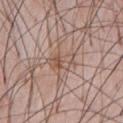Q: What did automated image analysis measure?
A: an eccentricity of roughly 0.9 and a symmetry-axis asymmetry near 0.35; a border-irregularity rating of about 4/10, a color-variation rating of about 7.5/10, and radial color variation of about 3; an automated nevus-likeness rating near 0 out of 100 and a lesion-detection confidence of about 100/100
Q: Lesion location?
A: the chest
Q: What is the lesion's diameter?
A: ≈4 mm
Q: What kind of image is this?
A: ~15 mm crop, total-body skin-cancer survey
Q: Patient demographics?
A: male, roughly 60 years of age